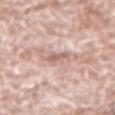Assessment:
The lesion was photographed on a routine skin check and not biopsied; there is no pathology result.
Clinical summary:
From the arm. The recorded lesion diameter is about 3 mm. A male patient in their mid- to late 70s. Captured under white-light illumination. A lesion tile, about 15 mm wide, cut from a 3D total-body photograph.